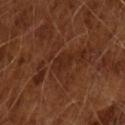Clinical impression:
Imaged during a routine full-body skin examination; the lesion was not biopsied and no histopathology is available.
Image and clinical context:
This image is a 15 mm lesion crop taken from a total-body photograph. This is a cross-polarized tile. Longest diameter approximately 2.5 mm. The patient is a male approximately 65 years of age.